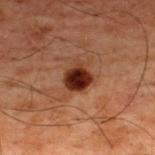This image is a 15 mm lesion crop taken from a total-body photograph. The recorded lesion diameter is about 3 mm. From the upper back. The subject is a male roughly 60 years of age.